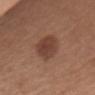Captured during whole-body skin photography for melanoma surveillance; the lesion was not biopsied.
The lesion is on the arm.
A female subject aged around 50.
Cropped from a total-body skin-imaging series; the visible field is about 15 mm.